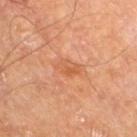Impression:
The lesion was tiled from a total-body skin photograph and was not biopsied.
Clinical summary:
A male subject aged approximately 65. Captured under cross-polarized illumination. The lesion is on the right thigh. The total-body-photography lesion software estimated a lesion area of about 4 mm² and an eccentricity of roughly 0.8. And it measured a lesion color around L≈55 a*≈26 b*≈38 in CIELAB, a lesion–skin lightness drop of about 7, and a normalized border contrast of about 5.5. The software also gave an automated nevus-likeness rating near 0 out of 100 and lesion-presence confidence of about 100/100. A lesion tile, about 15 mm wide, cut from a 3D total-body photograph. The recorded lesion diameter is about 3 mm.